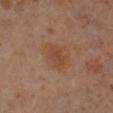| key | value |
|---|---|
| follow-up | catalogued during a skin exam; not biopsied |
| image | total-body-photography crop, ~15 mm field of view |
| subject | male, roughly 60 years of age |
| lesion size | ~3 mm (longest diameter) |
| location | the right lower leg |
| tile lighting | cross-polarized illumination |
| TBP lesion metrics | a border-irregularity index near 2/10, internal color variation of about 2 on a 0–10 scale, and peripheral color asymmetry of about 0.5 |